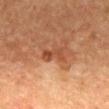The lesion was tiled from a total-body skin photograph and was not biopsied. From the mid back. Approximately 4 mm at its widest. A male patient aged approximately 50. Cropped from a total-body skin-imaging series; the visible field is about 15 mm. Imaged with cross-polarized lighting.